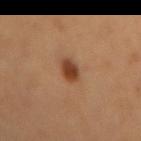Impression: This lesion was catalogued during total-body skin photography and was not selected for biopsy. Background: A region of skin cropped from a whole-body photographic capture, roughly 15 mm wide. On the mid back. The lesion's longest dimension is about 3 mm. Imaged with cross-polarized lighting. A female patient.